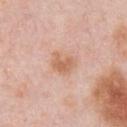Imaged during a routine full-body skin examination; the lesion was not biopsied and no histopathology is available. This is a white-light tile. The subject is a male aged around 60. A roughly 15 mm field-of-view crop from a total-body skin photograph. The lesion-visualizer software estimated a lesion area of about 6.5 mm², an outline eccentricity of about 0.5 (0 = round, 1 = elongated), and a shape-asymmetry score of about 0.25 (0 = symmetric). It also reported an average lesion color of about L≈64 a*≈21 b*≈33 (CIELAB) and a lesion-to-skin contrast of about 7 (normalized; higher = more distinct). It also reported a border-irregularity rating of about 2/10 and peripheral color asymmetry of about 1. On the front of the torso.